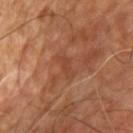Captured during whole-body skin photography for melanoma surveillance; the lesion was not biopsied.
The tile uses cross-polarized illumination.
An algorithmic analysis of the crop reported a footprint of about 3.5 mm² and a shape-asymmetry score of about 0.45 (0 = symmetric). The analysis additionally found a lesion color around L≈40 a*≈23 b*≈30 in CIELAB. It also reported a within-lesion color-variation index near 0/10 and peripheral color asymmetry of about 0. The analysis additionally found a lesion-detection confidence of about 100/100.
Located on the chest.
The subject is a male aged around 55.
A 15 mm crop from a total-body photograph taken for skin-cancer surveillance.
About 3 mm across.